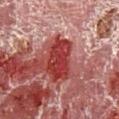The lesion was tiled from a total-body skin photograph and was not biopsied. The lesion-visualizer software estimated an area of roughly 12 mm² and a shape eccentricity near 0.85. The software also gave a normalized border contrast of about 11. The analysis additionally found border irregularity of about 2.5 on a 0–10 scale and a within-lesion color-variation index near 4.5/10. It also reported a detector confidence of about 55 out of 100 that the crop contains a lesion. A 15 mm close-up tile from a total-body photography series done for melanoma screening. A male subject, aged approximately 55. The lesion is located on the right lower leg. This is a cross-polarized tile. About 5.5 mm across.